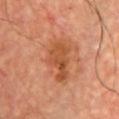Recorded during total-body skin imaging; not selected for excision or biopsy. On the chest. Automated tile analysis of the lesion measured a lesion area of about 11 mm², an eccentricity of roughly 0.85, and two-axis asymmetry of about 0.4. The software also gave a detector confidence of about 100 out of 100 that the crop contains a lesion. This image is a 15 mm lesion crop taken from a total-body photograph. The subject is a male about 65 years old. This is a cross-polarized tile.